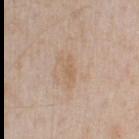The lesion was tiled from a total-body skin photograph and was not biopsied. A 15 mm crop from a total-body photograph taken for skin-cancer surveillance. Captured under white-light illumination. A male subject about 65 years old. Located on the arm. The lesion's longest dimension is about 3 mm. Automated tile analysis of the lesion measured an eccentricity of roughly 0.9 and two-axis asymmetry of about 0.4. It also reported an average lesion color of about L≈61 a*≈16 b*≈32 (CIELAB), about 6 CIELAB-L* units darker than the surrounding skin, and a lesion-to-skin contrast of about 5.5 (normalized; higher = more distinct). It also reported a border-irregularity rating of about 4/10, a within-lesion color-variation index near 0.5/10, and peripheral color asymmetry of about 0. It also reported a classifier nevus-likeness of about 0/100 and a detector confidence of about 100 out of 100 that the crop contains a lesion.